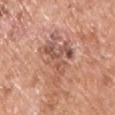Clinical impression:
This lesion was catalogued during total-body skin photography and was not selected for biopsy.
Background:
A lesion tile, about 15 mm wide, cut from a 3D total-body photograph. The patient is a male aged around 55. The lesion is on the front of the torso. The recorded lesion diameter is about 6 mm.